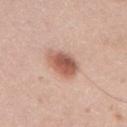Image and clinical context: Captured under white-light illumination. Longest diameter approximately 4 mm. This image is a 15 mm lesion crop taken from a total-body photograph. The total-body-photography lesion software estimated a border-irregularity index near 1.5/10, internal color variation of about 5.5 on a 0–10 scale, and radial color variation of about 2. And it measured lesion-presence confidence of about 100/100. The subject is a male aged 38 to 42. On the arm.